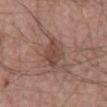Clinical impression: No biopsy was performed on this lesion — it was imaged during a full skin examination and was not determined to be concerning. Acquisition and patient details: A lesion tile, about 15 mm wide, cut from a 3D total-body photograph. Imaged with white-light lighting. The patient is a male aged approximately 55. Measured at roughly 3.5 mm in maximum diameter. The total-body-photography lesion software estimated border irregularity of about 3 on a 0–10 scale, internal color variation of about 3 on a 0–10 scale, and radial color variation of about 1. The analysis additionally found a classifier nevus-likeness of about 35/100. On the mid back.